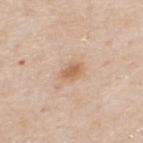Impression: Captured during whole-body skin photography for melanoma surveillance; the lesion was not biopsied. Image and clinical context: Longest diameter approximately 3 mm. This is a white-light tile. A male patient approximately 80 years of age. A 15 mm close-up tile from a total-body photography series done for melanoma screening. Located on the upper back.